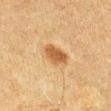Recorded during total-body skin imaging; not selected for excision or biopsy. Imaged with cross-polarized lighting. The lesion-visualizer software estimated an average lesion color of about L≈49 a*≈19 b*≈37 (CIELAB), about 11 CIELAB-L* units darker than the surrounding skin, and a normalized border contrast of about 8. It also reported border irregularity of about 2 on a 0–10 scale, internal color variation of about 2 on a 0–10 scale, and peripheral color asymmetry of about 1. It also reported a nevus-likeness score of about 90/100 and a detector confidence of about 100 out of 100 that the crop contains a lesion. A female subject aged 53 to 57. A 15 mm crop from a total-body photograph taken for skin-cancer surveillance. On the left thigh. Approximately 3 mm at its widest.